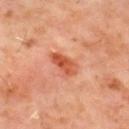{
  "biopsy_status": "not biopsied; imaged during a skin examination",
  "site": "back",
  "patient": {
    "sex": "male",
    "age_approx": 60
  },
  "automated_metrics": {
    "area_mm2_approx": 6.0,
    "eccentricity": 0.85,
    "cielab_L": 51,
    "cielab_a": 31,
    "cielab_b": 36,
    "vs_skin_darker_L": 11.0,
    "vs_skin_contrast_norm": 8.5,
    "border_irregularity_0_10": 2.5,
    "color_variation_0_10": 5.0,
    "peripheral_color_asymmetry": 2.0
  },
  "image": {
    "source": "total-body photography crop",
    "field_of_view_mm": 15
  },
  "lighting": "cross-polarized",
  "lesion_size": {
    "long_diameter_mm_approx": 3.5
  }
}